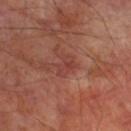Imaged during a routine full-body skin examination; the lesion was not biopsied and no histopathology is available. The total-body-photography lesion software estimated a lesion area of about 5 mm², a shape eccentricity near 0.7, and two-axis asymmetry of about 0.3. And it measured an average lesion color of about L≈40 a*≈26 b*≈26 (CIELAB), about 6 CIELAB-L* units darker than the surrounding skin, and a normalized lesion–skin contrast near 5.5. Captured under cross-polarized illumination. A male patient aged approximately 70. On the left lower leg. Longest diameter approximately 3 mm. Cropped from a whole-body photographic skin survey; the tile spans about 15 mm.